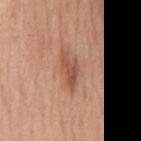Clinical impression: Part of a total-body skin-imaging series; this lesion was reviewed on a skin check and was not flagged for biopsy. Image and clinical context: The total-body-photography lesion software estimated a mean CIELAB color near L≈53 a*≈24 b*≈31 and about 10 CIELAB-L* units darker than the surrounding skin. It also reported border irregularity of about 4 on a 0–10 scale, a color-variation rating of about 2.5/10, and peripheral color asymmetry of about 0.5. Imaged with white-light lighting. The lesion is located on the mid back. A female patient about 65 years old. A lesion tile, about 15 mm wide, cut from a 3D total-body photograph. The recorded lesion diameter is about 4.5 mm.